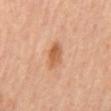  biopsy_status: not biopsied; imaged during a skin examination
  lesion_size:
    long_diameter_mm_approx: 3.0
  image:
    source: total-body photography crop
    field_of_view_mm: 15
  patient:
    sex: male
    age_approx: 55
  site: mid back